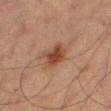Case summary:
• notes — no biopsy performed (imaged during a skin exam)
• imaging modality — total-body-photography crop, ~15 mm field of view
• subject — male, aged 63–67
• size — ~3.5 mm (longest diameter)
• lighting — cross-polarized illumination
• site — the leg